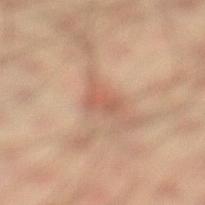Imaged during a routine full-body skin examination; the lesion was not biopsied and no histopathology is available.
The lesion is on the left lower leg.
The lesion-visualizer software estimated a border-irregularity rating of about 4/10, a within-lesion color-variation index near 3/10, and a peripheral color-asymmetry measure near 1. The analysis additionally found an automated nevus-likeness rating near 0 out of 100 and a detector confidence of about 100 out of 100 that the crop contains a lesion.
A region of skin cropped from a whole-body photographic capture, roughly 15 mm wide.
Imaged with cross-polarized lighting.
A male subject approximately 35 years of age.